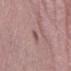No biopsy was performed on this lesion — it was imaged during a full skin examination and was not determined to be concerning.
A female subject aged approximately 40.
Located on the left lower leg.
A lesion tile, about 15 mm wide, cut from a 3D total-body photograph.
Imaged with white-light lighting.